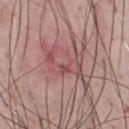No biopsy was performed on this lesion — it was imaged during a full skin examination and was not determined to be concerning. A close-up tile cropped from a whole-body skin photograph, about 15 mm across. The patient is a male approximately 50 years of age. On the front of the torso. Captured under white-light illumination.